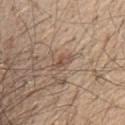| field | value |
|---|---|
| notes | imaged on a skin check; not biopsied |
| illumination | white-light |
| size | ≈2.5 mm |
| body site | the chest |
| subject | male, aged around 60 |
| acquisition | ~15 mm tile from a whole-body skin photo |